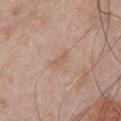Q: Was this lesion biopsied?
A: total-body-photography surveillance lesion; no biopsy
Q: Lesion location?
A: the chest
Q: What kind of image is this?
A: total-body-photography crop, ~15 mm field of view
Q: What did automated image analysis measure?
A: a lesion color around L≈58 a*≈18 b*≈28 in CIELAB, about 6 CIELAB-L* units darker than the surrounding skin, and a lesion-to-skin contrast of about 4.5 (normalized; higher = more distinct); border irregularity of about 4 on a 0–10 scale, a within-lesion color-variation index near 0/10, and a peripheral color-asymmetry measure near 0; a nevus-likeness score of about 0/100 and a detector confidence of about 100 out of 100 that the crop contains a lesion
Q: Illumination type?
A: white-light
Q: What is the lesion's diameter?
A: ~2.5 mm (longest diameter)
Q: What are the patient's age and sex?
A: male, aged approximately 55